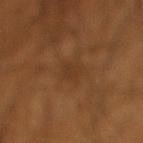Captured during whole-body skin photography for melanoma surveillance; the lesion was not biopsied. A 15 mm close-up tile from a total-body photography series done for melanoma screening. A male patient, aged 53 to 57. From the right lower leg.